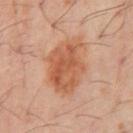No biopsy was performed on this lesion — it was imaged during a full skin examination and was not determined to be concerning. The lesion is located on the front of the torso. Imaged with cross-polarized lighting. A male patient aged around 60. A 15 mm crop from a total-body photograph taken for skin-cancer surveillance. Measured at roughly 6.5 mm in maximum diameter.